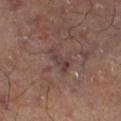Q: Was this lesion biopsied?
A: catalogued during a skin exam; not biopsied
Q: How was the tile lit?
A: cross-polarized
Q: Lesion location?
A: the left thigh
Q: How was this image acquired?
A: 15 mm crop, total-body photography